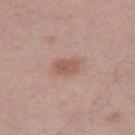No biopsy was performed on this lesion — it was imaged during a full skin examination and was not determined to be concerning.
A 15 mm crop from a total-body photograph taken for skin-cancer surveillance.
About 3.5 mm across.
A male subject, aged 43 to 47.
The lesion-visualizer software estimated a lesion area of about 5 mm² and a symmetry-axis asymmetry near 0.15. The software also gave a mean CIELAB color near L≈56 a*≈21 b*≈26 and a lesion-to-skin contrast of about 6.5 (normalized; higher = more distinct). The analysis additionally found border irregularity of about 1.5 on a 0–10 scale, internal color variation of about 2.5 on a 0–10 scale, and radial color variation of about 1.
The tile uses white-light illumination.
On the right thigh.